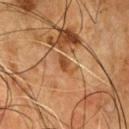biopsy_status: not biopsied; imaged during a skin examination